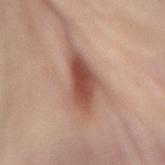The lesion was tiled from a total-body skin photograph and was not biopsied. The total-body-photography lesion software estimated an area of roughly 12 mm², an outline eccentricity of about 0.85 (0 = round, 1 = elongated), and a symmetry-axis asymmetry near 0.35. The software also gave border irregularity of about 3.5 on a 0–10 scale, a color-variation rating of about 6/10, and radial color variation of about 2. The lesion is on the abdomen. Cropped from a total-body skin-imaging series; the visible field is about 15 mm. Measured at roughly 6 mm in maximum diameter. A female subject, about 40 years old.